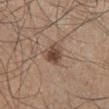{"biopsy_status": "not biopsied; imaged during a skin examination", "image": {"source": "total-body photography crop", "field_of_view_mm": 15}, "lesion_size": {"long_diameter_mm_approx": 3.0}, "site": "right lower leg", "lighting": "white-light", "automated_metrics": {"area_mm2_approx": 5.5, "eccentricity": 0.65, "shape_asymmetry": 0.2, "cielab_L": 45, "cielab_a": 18, "cielab_b": 26, "vs_skin_darker_L": 12.0, "vs_skin_contrast_norm": 9.0, "border_irregularity_0_10": 1.5, "color_variation_0_10": 5.0, "peripheral_color_asymmetry": 1.5, "nevus_likeness_0_100": 95, "lesion_detection_confidence_0_100": 100}, "patient": {"sex": "male", "age_approx": 45}}